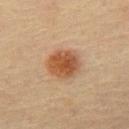Assessment: The lesion was tiled from a total-body skin photograph and was not biopsied. Context: A male subject aged 63–67. A region of skin cropped from a whole-body photographic capture, roughly 15 mm wide. Measured at roughly 4 mm in maximum diameter. The lesion is located on the front of the torso. The lesion-visualizer software estimated a lesion area of about 12 mm², an outline eccentricity of about 0.4 (0 = round, 1 = elongated), and two-axis asymmetry of about 0.15. The software also gave a nevus-likeness score of about 100/100 and a detector confidence of about 100 out of 100 that the crop contains a lesion. Imaged with cross-polarized lighting.